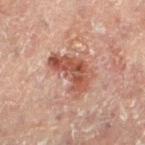workup: no biopsy performed (imaged during a skin exam)
lesion size: ≈5.5 mm
acquisition: ~15 mm tile from a whole-body skin photo
image-analysis metrics: a border-irregularity index near 5.5/10, a within-lesion color-variation index near 5/10, and peripheral color asymmetry of about 1.5
site: the right leg
subject: female, aged 78 to 82